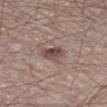notes=catalogued during a skin exam; not biopsied
subject=male, in their mid- to late 70s
size=about 3 mm
lighting=white-light
body site=the right lower leg
image-analysis metrics=a shape eccentricity near 0.7 and a symmetry-axis asymmetry near 0.3; an automated nevus-likeness rating near 65 out of 100 and a detector confidence of about 100 out of 100 that the crop contains a lesion
image source=~15 mm crop, total-body skin-cancer survey